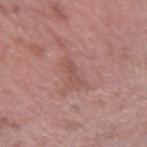biopsy status = catalogued during a skin exam; not biopsied | image source = ~15 mm tile from a whole-body skin photo | patient = male, approximately 40 years of age | automated metrics = roughly 7 lightness units darker than nearby skin and a normalized lesion–skin contrast near 5; a border-irregularity rating of about 6/10 and a within-lesion color-variation index near 0/10; a nevus-likeness score of about 0/100 and a detector confidence of about 70 out of 100 that the crop contains a lesion | anatomic site = the right upper arm | size = ≈3.5 mm | lighting = white-light illumination.